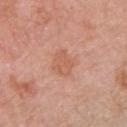acquisition: ~15 mm tile from a whole-body skin photo | tile lighting: white-light | TBP lesion metrics: a lesion color around L≈60 a*≈24 b*≈32 in CIELAB and a lesion-to-skin contrast of about 5 (normalized; higher = more distinct); a classifier nevus-likeness of about 5/100 | subject: female, roughly 60 years of age | lesion size: about 3 mm | anatomic site: the left upper arm.